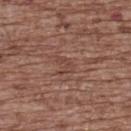Q: What is the anatomic site?
A: the upper back
Q: What are the patient's age and sex?
A: female, aged approximately 75
Q: How was this image acquired?
A: 15 mm crop, total-body photography